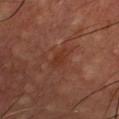{
  "biopsy_status": "not biopsied; imaged during a skin examination",
  "image": {
    "source": "total-body photography crop",
    "field_of_view_mm": 15
  },
  "patient": {
    "sex": "male",
    "age_approx": 55
  },
  "site": "chest"
}